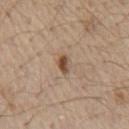<tbp_lesion>
  <biopsy_status>not biopsied; imaged during a skin examination</biopsy_status>
  <patient>
    <sex>male</sex>
    <age_approx>70</age_approx>
  </patient>
  <image>
    <source>total-body photography crop</source>
    <field_of_view_mm>15</field_of_view_mm>
  </image>
  <automated_metrics>
    <border_irregularity_0_10>3.0</border_irregularity_0_10>
    <peripheral_color_asymmetry>1.0</peripheral_color_asymmetry>
    <nevus_likeness_0_100>90</nevus_likeness_0_100>
  </automated_metrics>
  <lighting>white-light</lighting>
  <site>chest</site>
  <lesion_size>
    <long_diameter_mm_approx>2.5</long_diameter_mm_approx>
  </lesion_size>
</tbp_lesion>